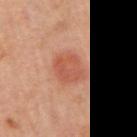| feature | finding |
|---|---|
| biopsy status | imaged on a skin check; not biopsied |
| acquisition | ~15 mm tile from a whole-body skin photo |
| body site | the right upper arm |
| patient | female, approximately 45 years of age |
| lighting | cross-polarized illumination |